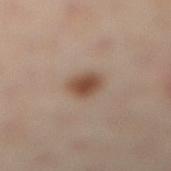• follow-up — catalogued during a skin exam; not biopsied
• subject — female, approximately 40 years of age
• location — the left leg
• imaging modality — ~15 mm tile from a whole-body skin photo
• TBP lesion metrics — an average lesion color of about L≈50 a*≈18 b*≈28 (CIELAB); a border-irregularity rating of about 1.5/10, a within-lesion color-variation index near 3/10, and a peripheral color-asymmetry measure near 0.5; a lesion-detection confidence of about 100/100
• illumination — cross-polarized